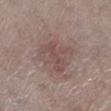- notes — total-body-photography surveillance lesion; no biopsy
- diameter — ~4.5 mm (longest diameter)
- anatomic site — the leg
- automated metrics — an average lesion color of about L≈49 a*≈17 b*≈19 (CIELAB), roughly 7 lightness units darker than nearby skin, and a normalized lesion–skin contrast near 5.5
- patient — male, aged 78–82
- tile lighting — white-light
- acquisition — 15 mm crop, total-body photography